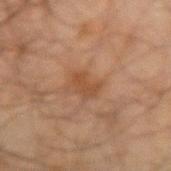Assessment:
Part of a total-body skin-imaging series; this lesion was reviewed on a skin check and was not flagged for biopsy.
Clinical summary:
The lesion-visualizer software estimated a normalized lesion–skin contrast near 6. The analysis additionally found a nevus-likeness score of about 0/100 and a detector confidence of about 100 out of 100 that the crop contains a lesion. A male patient, aged around 70. The lesion is on the arm. About 2.5 mm across. Cropped from a whole-body photographic skin survey; the tile spans about 15 mm.